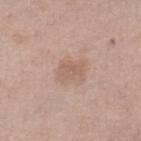Assessment: The lesion was tiled from a total-body skin photograph and was not biopsied. Image and clinical context: From the right thigh. The recorded lesion diameter is about 3 mm. A region of skin cropped from a whole-body photographic capture, roughly 15 mm wide. The total-body-photography lesion software estimated an eccentricity of roughly 0.8. The analysis additionally found a lesion–skin lightness drop of about 7 and a normalized border contrast of about 5. It also reported a lesion-detection confidence of about 100/100. A female patient, roughly 65 years of age.